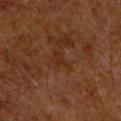Clinical summary:
Longest diameter approximately 3 mm. The patient is a male aged 58–62. Automated image analysis of the tile measured a footprint of about 3.5 mm², an eccentricity of roughly 0.9, and a shape-asymmetry score of about 0.5 (0 = symmetric). It also reported a border-irregularity rating of about 5/10, a within-lesion color-variation index near 1.5/10, and peripheral color asymmetry of about 0.5. A lesion tile, about 15 mm wide, cut from a 3D total-body photograph. The lesion is located on the front of the torso.